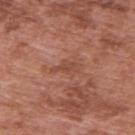From the upper back.
A male patient, about 70 years old.
A region of skin cropped from a whole-body photographic capture, roughly 15 mm wide.
The tile uses white-light illumination.
The total-body-photography lesion software estimated a lesion area of about 3 mm², an outline eccentricity of about 0.85 (0 = round, 1 = elongated), and a shape-asymmetry score of about 0.4 (0 = symmetric). It also reported a mean CIELAB color near L≈47 a*≈25 b*≈29, a lesion–skin lightness drop of about 7, and a normalized border contrast of about 5.5.
The lesion's longest dimension is about 3 mm.